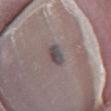| key | value |
|---|---|
| acquisition | ~15 mm tile from a whole-body skin photo |
| lesion diameter | ≈2.5 mm |
| image-analysis metrics | an average lesion color of about L≈45 a*≈9 b*≈11 (CIELAB), about 11 CIELAB-L* units darker than the surrounding skin, and a lesion-to-skin contrast of about 9 (normalized; higher = more distinct) |
| anatomic site | the right lower leg |
| illumination | white-light |
| patient | male, about 45 years old |